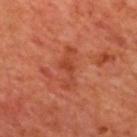{
  "biopsy_status": "not biopsied; imaged during a skin examination",
  "patient": {
    "sex": "male",
    "age_approx": 70
  },
  "image": {
    "source": "total-body photography crop",
    "field_of_view_mm": 15
  },
  "site": "upper back"
}